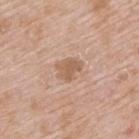Q: Is there a histopathology result?
A: imaged on a skin check; not biopsied
Q: What kind of image is this?
A: 15 mm crop, total-body photography
Q: Illumination type?
A: white-light illumination
Q: What is the lesion's diameter?
A: ~3 mm (longest diameter)
Q: Where on the body is the lesion?
A: the upper back
Q: Patient demographics?
A: male, about 65 years old